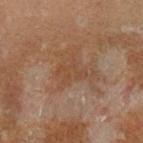<tbp_lesion>
<biopsy_status>not biopsied; imaged during a skin examination</biopsy_status>
<lesion_size>
  <long_diameter_mm_approx>4.0</long_diameter_mm_approx>
</lesion_size>
<image>
  <source>total-body photography crop</source>
  <field_of_view_mm>15</field_of_view_mm>
</image>
<site>left lower leg</site>
<lighting>cross-polarized</lighting>
<patient>
  <sex>male</sex>
  <age_approx>30</age_approx>
</patient>
</tbp_lesion>